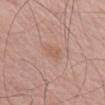Imaged during a routine full-body skin examination; the lesion was not biopsied and no histopathology is available.
The total-body-photography lesion software estimated a lesion color around L≈59 a*≈20 b*≈28 in CIELAB, roughly 5 lightness units darker than nearby skin, and a normalized border contrast of about 4.5.
A male patient, in their mid- to late 60s.
Located on the right upper arm.
A lesion tile, about 15 mm wide, cut from a 3D total-body photograph.
Imaged with white-light lighting.
About 2.5 mm across.